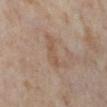Recorded during total-body skin imaging; not selected for excision or biopsy. This is a cross-polarized tile. A 15 mm close-up extracted from a 3D total-body photography capture. Located on the left lower leg. A female patient approximately 55 years of age.